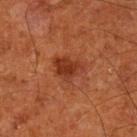The lesion was photographed on a routine skin check and not biopsied; there is no pathology result.
Measured at roughly 4 mm in maximum diameter.
Automated tile analysis of the lesion measured a lesion area of about 8 mm², a shape eccentricity near 0.65, and a shape-asymmetry score of about 0.4 (0 = symmetric). The analysis additionally found internal color variation of about 3.5 on a 0–10 scale and radial color variation of about 1.5.
A close-up tile cropped from a whole-body skin photograph, about 15 mm across.
The lesion is located on the left lower leg.
A male subject in their mid-60s.